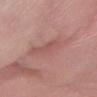The lesion was photographed on a routine skin check and not biopsied; there is no pathology result.
The subject is a female aged 73–77.
This is a white-light tile.
The lesion's longest dimension is about 5.5 mm.
The lesion is on the arm.
A 15 mm close-up extracted from a 3D total-body photography capture.